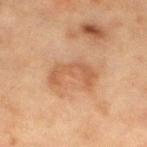Acquisition and patient details: The subject is a female in their mid-50s. The lesion is located on the left lower leg. A close-up tile cropped from a whole-body skin photograph, about 15 mm across.